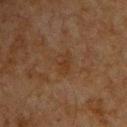site: the chest | acquisition: 15 mm crop, total-body photography | patient: male, approximately 65 years of age | lesion size: ~2.5 mm (longest diameter).